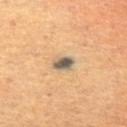Findings:
• biopsy status — catalogued during a skin exam; not biopsied
• lighting — cross-polarized illumination
• acquisition — ~15 mm tile from a whole-body skin photo
• TBP lesion metrics — an area of roughly 5 mm² and two-axis asymmetry of about 0.25; a nevus-likeness score of about 100/100
• patient — female, approximately 50 years of age
• lesion diameter — ~3 mm (longest diameter)